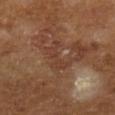Notes:
– workup · no biopsy performed (imaged during a skin exam)
– patient · male, aged approximately 65
– image source · ~15 mm crop, total-body skin-cancer survey
– location · the left lower leg
– lighting · cross-polarized illumination